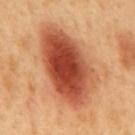Q: Was this lesion biopsied?
A: no biopsy performed (imaged during a skin exam)
Q: Illumination type?
A: cross-polarized
Q: What is the imaging modality?
A: total-body-photography crop, ~15 mm field of view
Q: Lesion size?
A: about 10.5 mm
Q: What are the patient's age and sex?
A: male, approximately 50 years of age
Q: Lesion location?
A: the mid back